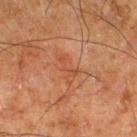No biopsy was performed on this lesion — it was imaged during a full skin examination and was not determined to be concerning. This is a cross-polarized tile. From the right thigh. A 15 mm crop from a total-body photograph taken for skin-cancer surveillance. The total-body-photography lesion software estimated an average lesion color of about L≈39 a*≈22 b*≈29 (CIELAB), roughly 5 lightness units darker than nearby skin, and a lesion-to-skin contrast of about 5 (normalized; higher = more distinct). The software also gave an automated nevus-likeness rating near 0 out of 100 and a detector confidence of about 100 out of 100 that the crop contains a lesion. The subject is a male roughly 80 years of age.